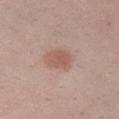{
  "lesion_size": {
    "long_diameter_mm_approx": 3.0
  },
  "image": {
    "source": "total-body photography crop",
    "field_of_view_mm": 15
  },
  "lighting": "white-light",
  "automated_metrics": {
    "vs_skin_darker_L": 9.0,
    "vs_skin_contrast_norm": 6.5
  },
  "site": "right upper arm",
  "patient": {
    "sex": "male",
    "age_approx": 50
  }
}